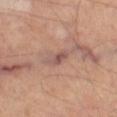The lesion was tiled from a total-body skin photograph and was not biopsied. The tile uses cross-polarized illumination. On the leg. About 3 mm across. A male subject, about 65 years old. A 15 mm close-up extracted from a 3D total-body photography capture.